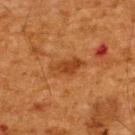imaging modality — ~15 mm crop, total-body skin-cancer survey
lighting — cross-polarized illumination
subject — male, aged approximately 65
body site — the upper back
lesion diameter — about 4.5 mm